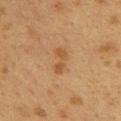The lesion was photographed on a routine skin check and not biopsied; there is no pathology result.